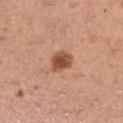No biopsy was performed on this lesion — it was imaged during a full skin examination and was not determined to be concerning. The lesion is on the left lower leg. A 15 mm crop from a total-body photograph taken for skin-cancer surveillance. A female subject, aged 28–32.